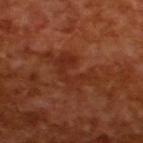Assessment:
The lesion was photographed on a routine skin check and not biopsied; there is no pathology result.
Context:
A male subject approximately 65 years of age. This image is a 15 mm lesion crop taken from a total-body photograph.